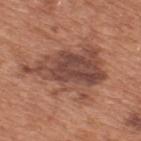The lesion was photographed on a routine skin check and not biopsied; there is no pathology result. This image is a 15 mm lesion crop taken from a total-body photograph. The lesion is located on the upper back. A male patient roughly 65 years of age.